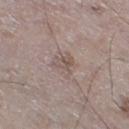workup: total-body-photography surveillance lesion; no biopsy
lesion diameter: ≈3.5 mm
image-analysis metrics: an eccentricity of roughly 0.55 and a symmetry-axis asymmetry near 0.35; a mean CIELAB color near L≈54 a*≈13 b*≈20 and a lesion–skin lightness drop of about 7
image source: ~15 mm tile from a whole-body skin photo
lighting: white-light illumination
subject: male, aged 63 to 67
anatomic site: the right thigh